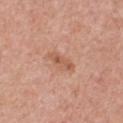<record>
<biopsy_status>not biopsied; imaged during a skin examination</biopsy_status>
<automated_metrics>
  <area_mm2_approx>4.0</area_mm2_approx>
  <lesion_detection_confidence_0_100>100</lesion_detection_confidence_0_100>
</automated_metrics>
<lighting>white-light</lighting>
<site>chest</site>
<image>
  <source>total-body photography crop</source>
  <field_of_view_mm>15</field_of_view_mm>
</image>
<lesion_size>
  <long_diameter_mm_approx>3.5</long_diameter_mm_approx>
</lesion_size>
<patient>
  <sex>female</sex>
  <age_approx>40</age_approx>
</patient>
</record>